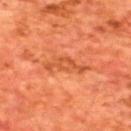{
  "biopsy_status": "not biopsied; imaged during a skin examination",
  "image": {
    "source": "total-body photography crop",
    "field_of_view_mm": 15
  },
  "patient": {
    "sex": "male",
    "age_approx": 65
  },
  "site": "upper back",
  "lighting": "cross-polarized"
}